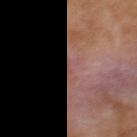No biopsy was performed on this lesion — it was imaged during a full skin examination and was not determined to be concerning. The recorded lesion diameter is about 1.5 mm. On the upper back. The lesion-visualizer software estimated a lesion area of about 0.5 mm², an eccentricity of roughly 0.95, and two-axis asymmetry of about 0.4. It also reported a lesion color around L≈47 a*≈22 b*≈23 in CIELAB, roughly 4 lightness units darker than nearby skin, and a lesion-to-skin contrast of about 3 (normalized; higher = more distinct). And it measured a border-irregularity index near 4.5/10, internal color variation of about 0 on a 0–10 scale, and peripheral color asymmetry of about 0. It also reported a classifier nevus-likeness of about 0/100 and a lesion-detection confidence of about 85/100. A 15 mm crop from a total-body photograph taken for skin-cancer surveillance. A female patient, roughly 50 years of age.